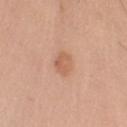Clinical impression: Part of a total-body skin-imaging series; this lesion was reviewed on a skin check and was not flagged for biopsy. Clinical summary: Imaged with white-light lighting. A male subject about 70 years old. A 15 mm close-up tile from a total-body photography series done for melanoma screening. Measured at roughly 3 mm in maximum diameter. On the arm.